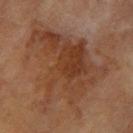Assessment: The lesion was photographed on a routine skin check and not biopsied; there is no pathology result. Background: Imaged with cross-polarized lighting. A region of skin cropped from a whole-body photographic capture, roughly 15 mm wide. The lesion-visualizer software estimated a footprint of about 65 mm², an eccentricity of roughly 0.35, and a shape-asymmetry score of about 0.45 (0 = symmetric). It also reported an automated nevus-likeness rating near 10 out of 100. A female subject, aged approximately 60. From the left forearm.